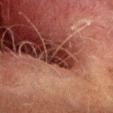follow-up: total-body-photography surveillance lesion; no biopsy.